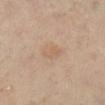Q: Was a biopsy performed?
A: total-body-photography surveillance lesion; no biopsy
Q: Patient demographics?
A: female, aged 38–42
Q: What did automated image analysis measure?
A: a lesion area of about 3 mm², an outline eccentricity of about 0.9 (0 = round, 1 = elongated), and a symmetry-axis asymmetry near 0.35; border irregularity of about 3.5 on a 0–10 scale, internal color variation of about 0.5 on a 0–10 scale, and radial color variation of about 0
Q: What is the anatomic site?
A: the right leg
Q: What is the lesion's diameter?
A: ≈3 mm
Q: What kind of image is this?
A: ~15 mm tile from a whole-body skin photo
Q: How was the tile lit?
A: cross-polarized